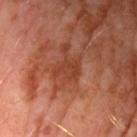<tbp_lesion>
  <biopsy_status>not biopsied; imaged during a skin examination</biopsy_status>
  <patient>
    <sex>male</sex>
    <age_approx>60</age_approx>
  </patient>
  <automated_metrics>
    <cielab_L>38</cielab_L>
    <cielab_a>27</cielab_a>
    <cielab_b>31</cielab_b>
    <vs_skin_darker_L>7.0</vs_skin_darker_L>
    <vs_skin_contrast_norm>6.0</vs_skin_contrast_norm>
    <peripheral_color_asymmetry>0.5</peripheral_color_asymmetry>
    <nevus_likeness_0_100>0</nevus_likeness_0_100>
    <lesion_detection_confidence_0_100>100</lesion_detection_confidence_0_100>
  </automated_metrics>
  <image>
    <source>total-body photography crop</source>
    <field_of_view_mm>15</field_of_view_mm>
  </image>
  <site>left upper arm</site>
</tbp_lesion>